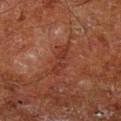Notes:
• follow-up · no biopsy performed (imaged during a skin exam)
• tile lighting · cross-polarized
• image · total-body-photography crop, ~15 mm field of view
• body site · the left lower leg
• patient · male, aged 63 to 67